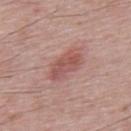Captured during whole-body skin photography for melanoma surveillance; the lesion was not biopsied.
Captured under white-light illumination.
Located on the upper back.
Approximately 4.5 mm at its widest.
A male subject, approximately 65 years of age.
A region of skin cropped from a whole-body photographic capture, roughly 15 mm wide.